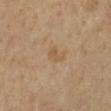The lesion was tiled from a total-body skin photograph and was not biopsied. A male subject, in their mid- to late 60s. The total-body-photography lesion software estimated a mean CIELAB color near L≈55 a*≈15 b*≈34. The software also gave a classifier nevus-likeness of about 0/100. The lesion is located on the left lower leg. A roughly 15 mm field-of-view crop from a total-body skin photograph.